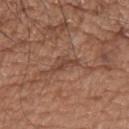• workup: imaged on a skin check; not biopsied
• acquisition: ~15 mm crop, total-body skin-cancer survey
• tile lighting: white-light illumination
• automated metrics: a shape eccentricity near 0.9 and a shape-asymmetry score of about 0.55 (0 = symmetric); an average lesion color of about L≈44 a*≈21 b*≈27 (CIELAB); a classifier nevus-likeness of about 0/100 and a lesion-detection confidence of about 65/100
• site: the upper back
• lesion size: about 3.5 mm
• patient: male, approximately 65 years of age